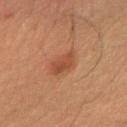Impression: This lesion was catalogued during total-body skin photography and was not selected for biopsy. Image and clinical context: From the right forearm. A male subject in their mid-30s. About 3 mm across. Imaged with cross-polarized lighting. This image is a 15 mm lesion crop taken from a total-body photograph.